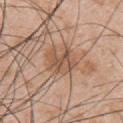Findings:
– follow-up · imaged on a skin check; not biopsied
– lesion size · ~4 mm (longest diameter)
– subject · male, in their mid-50s
– imaging modality · ~15 mm crop, total-body skin-cancer survey
– body site · the left upper arm